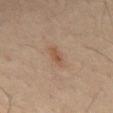Case summary:
- follow-up · imaged on a skin check; not biopsied
- automated metrics · a lesion–skin lightness drop of about 7; a nevus-likeness score of about 45/100 and a lesion-detection confidence of about 100/100
- anatomic site · the abdomen
- image source · total-body-photography crop, ~15 mm field of view
- patient · male, approximately 65 years of age
- lesion size · ≈3 mm